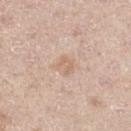Captured during whole-body skin photography for melanoma surveillance; the lesion was not biopsied. Cropped from a total-body skin-imaging series; the visible field is about 15 mm. On the left thigh. A male patient, about 65 years old.